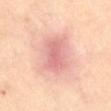Recorded during total-body skin imaging; not selected for excision or biopsy. From the abdomen. The tile uses cross-polarized illumination. The patient is a female approximately 60 years of age. The lesion's longest dimension is about 5 mm. The total-body-photography lesion software estimated an area of roughly 11 mm² and a shape-asymmetry score of about 0.2 (0 = symmetric). And it measured a mean CIELAB color near L≈69 a*≈28 b*≈25, roughly 10 lightness units darker than nearby skin, and a normalized border contrast of about 6. The analysis additionally found a within-lesion color-variation index near 2.5/10 and peripheral color asymmetry of about 0.5. A 15 mm crop from a total-body photograph taken for skin-cancer surveillance.